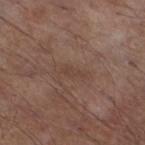Q: What is the anatomic site?
A: the leg
Q: What is the imaging modality?
A: total-body-photography crop, ~15 mm field of view
Q: How was the tile lit?
A: white-light illumination
Q: What is the lesion's diameter?
A: about 2.5 mm
Q: Who is the patient?
A: male, in their mid- to late 50s
Q: Automated lesion metrics?
A: an area of roughly 2 mm², an outline eccentricity of about 0.95 (0 = round, 1 = elongated), and two-axis asymmetry of about 0.55; an average lesion color of about L≈41 a*≈18 b*≈23 (CIELAB) and roughly 5 lightness units darker than nearby skin; a border-irregularity rating of about 6.5/10, a within-lesion color-variation index near 0/10, and peripheral color asymmetry of about 0